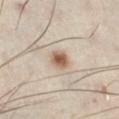* notes — no biopsy performed (imaged during a skin exam)
* lesion size — ≈3 mm
* lighting — cross-polarized
* anatomic site — the left lower leg
* imaging modality — 15 mm crop, total-body photography
* subject — male, aged 48 to 52